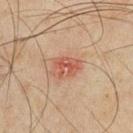biopsy status: no biopsy performed (imaged during a skin exam)
diameter: ≈3.5 mm
site: the front of the torso
subject: male, in their mid-40s
imaging modality: 15 mm crop, total-body photography